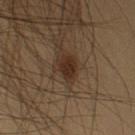{"lighting": "cross-polarized", "image": {"source": "total-body photography crop", "field_of_view_mm": 15}, "automated_metrics": {"area_mm2_approx": 4.0, "shape_asymmetry": 0.25, "cielab_L": 28, "cielab_a": 16, "cielab_b": 25, "vs_skin_contrast_norm": 10.0, "border_irregularity_0_10": 2.0, "color_variation_0_10": 2.5, "peripheral_color_asymmetry": 1.0, "nevus_likeness_0_100": 90, "lesion_detection_confidence_0_100": 100}, "site": "left upper arm", "patient": {"sex": "male", "age_approx": 40}, "lesion_size": {"long_diameter_mm_approx": 2.5}}